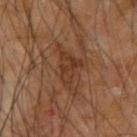{"biopsy_status": "not biopsied; imaged during a skin examination", "lighting": "cross-polarized", "site": "right upper arm", "automated_metrics": {"border_irregularity_0_10": 8.0, "color_variation_0_10": 3.0, "peripheral_color_asymmetry": 1.0}, "patient": {"sex": "male", "age_approx": 65}, "image": {"source": "total-body photography crop", "field_of_view_mm": 15}}